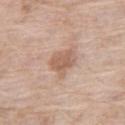Q: Was this lesion biopsied?
A: imaged on a skin check; not biopsied
Q: Who is the patient?
A: female, approximately 75 years of age
Q: What is the lesion's diameter?
A: about 3.5 mm
Q: What is the imaging modality?
A: 15 mm crop, total-body photography
Q: What did automated image analysis measure?
A: an average lesion color of about L≈59 a*≈19 b*≈29 (CIELAB), a lesion–skin lightness drop of about 10, and a normalized border contrast of about 6.5; a classifier nevus-likeness of about 15/100 and lesion-presence confidence of about 100/100
Q: Lesion location?
A: the right thigh
Q: How was the tile lit?
A: white-light illumination